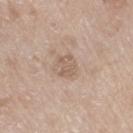Located on the right thigh.
The subject is a female about 75 years old.
Cropped from a total-body skin-imaging series; the visible field is about 15 mm.